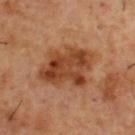Clinical impression:
Recorded during total-body skin imaging; not selected for excision or biopsy.
Acquisition and patient details:
The subject is a male aged approximately 55. A 15 mm close-up tile from a total-body photography series done for melanoma screening. This is a cross-polarized tile. Located on the upper back.